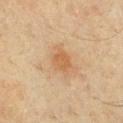Notes:
- follow-up: no biopsy performed (imaged during a skin exam)
- patient: male, aged around 40
- illumination: cross-polarized
- automated metrics: a footprint of about 5 mm², an outline eccentricity of about 0.6 (0 = round, 1 = elongated), and two-axis asymmetry of about 0.2; a lesion color around L≈53 a*≈19 b*≈35 in CIELAB; a nevus-likeness score of about 20/100 and lesion-presence confidence of about 100/100
- diameter: ≈3 mm
- body site: the front of the torso
- acquisition: ~15 mm crop, total-body skin-cancer survey